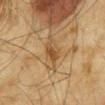Part of a total-body skin-imaging series; this lesion was reviewed on a skin check and was not flagged for biopsy. Captured under cross-polarized illumination. The total-body-photography lesion software estimated a lesion area of about 4.5 mm², an outline eccentricity of about 0.8 (0 = round, 1 = elongated), and a symmetry-axis asymmetry near 0.25. It also reported roughly 9 lightness units darker than nearby skin and a normalized border contrast of about 6.5. The software also gave border irregularity of about 2.5 on a 0–10 scale and radial color variation of about 1. The subject is a male roughly 65 years of age. About 3 mm across. A 15 mm crop from a total-body photograph taken for skin-cancer surveillance. The lesion is on the back.